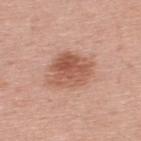Notes:
• subject: male, aged 63–67
• location: the upper back
• image: total-body-photography crop, ~15 mm field of view
• illumination: white-light